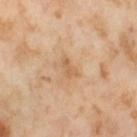Acquisition and patient details:
Longest diameter approximately 2.5 mm. The tile uses cross-polarized illumination. Located on the left thigh. A 15 mm close-up tile from a total-body photography series done for melanoma screening. The patient is a female in their mid-50s.